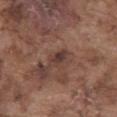biopsy status: catalogued during a skin exam; not biopsied
image: ~15 mm crop, total-body skin-cancer survey
patient: male, about 75 years old
tile lighting: white-light
size: about 3 mm
site: the abdomen
automated metrics: a footprint of about 4 mm², an outline eccentricity of about 0.85 (0 = round, 1 = elongated), and two-axis asymmetry of about 0.2; a mean CIELAB color near L≈37 a*≈18 b*≈22 and a lesion–skin lightness drop of about 9